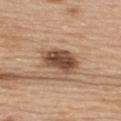The lesion was photographed on a routine skin check and not biopsied; there is no pathology result. Measured at roughly 4.5 mm in maximum diameter. A 15 mm close-up extracted from a 3D total-body photography capture. Located on the upper back. Captured under white-light illumination. A female subject, approximately 65 years of age.